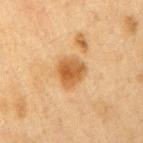  biopsy_status: not biopsied; imaged during a skin examination
  lesion_size:
    long_diameter_mm_approx: 3.5
  automated_metrics:
    area_mm2_approx: 8.5
    eccentricity: 0.3
    shape_asymmetry: 0.25
    cielab_L: 51
    cielab_a: 20
    cielab_b: 39
    vs_skin_darker_L: 11.0
    vs_skin_contrast_norm: 8.5
    border_irregularity_0_10: 2.0
    color_variation_0_10: 4.5
    peripheral_color_asymmetry: 1.5
  lighting: cross-polarized
  patient:
    sex: female
    age_approx: 40
  site: right upper arm
  image:
    source: total-body photography crop
    field_of_view_mm: 15